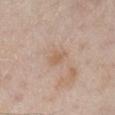Impression: This lesion was catalogued during total-body skin photography and was not selected for biopsy. Acquisition and patient details: An algorithmic analysis of the crop reported a shape eccentricity near 0.7 and a symmetry-axis asymmetry near 0.25. The software also gave a mean CIELAB color near L≈60 a*≈17 b*≈31 and a lesion–skin lightness drop of about 7. A 15 mm crop from a total-body photograph taken for skin-cancer surveillance. The recorded lesion diameter is about 2.5 mm. The tile uses white-light illumination. The subject is a male approximately 60 years of age. The lesion is located on the leg.